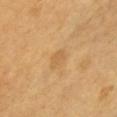Q: Was this lesion biopsied?
A: imaged on a skin check; not biopsied
Q: Lesion location?
A: the chest
Q: How was this image acquired?
A: 15 mm crop, total-body photography
Q: Patient demographics?
A: male, roughly 60 years of age